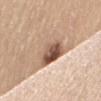* notes: no biopsy performed (imaged during a skin exam)
* image-analysis metrics: a lesion color around L≈60 a*≈17 b*≈29 in CIELAB, about 13 CIELAB-L* units darker than the surrounding skin, and a normalized lesion–skin contrast near 8.5; a nevus-likeness score of about 60/100
* imaging modality: 15 mm crop, total-body photography
* body site: the mid back
* size: about 7 mm
* subject: female, aged around 65